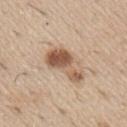{
  "biopsy_status": "not biopsied; imaged during a skin examination",
  "image": {
    "source": "total-body photography crop",
    "field_of_view_mm": 15
  },
  "lesion_size": {
    "long_diameter_mm_approx": 5.5
  },
  "automated_metrics": {
    "cielab_L": 56,
    "cielab_a": 19,
    "cielab_b": 31,
    "vs_skin_darker_L": 14.0,
    "vs_skin_contrast_norm": 9.5,
    "nevus_likeness_0_100": 80
  },
  "patient": {
    "sex": "male",
    "age_approx": 60
  },
  "site": "right upper arm"
}